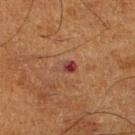- workup · no biopsy performed (imaged during a skin exam)
- imaging modality · ~15 mm tile from a whole-body skin photo
- size · about 2.5 mm
- site · the right lower leg
- tile lighting · cross-polarized illumination
- subject · male, aged 63–67